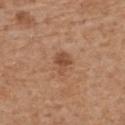Impression: This lesion was catalogued during total-body skin photography and was not selected for biopsy. Context: A 15 mm crop from a total-body photograph taken for skin-cancer surveillance. The lesion is located on the upper back. Measured at roughly 2.5 mm in maximum diameter. This is a white-light tile. A male patient, roughly 70 years of age.